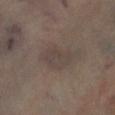This lesion was catalogued during total-body skin photography and was not selected for biopsy.
A male subject about 65 years old.
The lesion is located on the leg.
This image is a 15 mm lesion crop taken from a total-body photograph.